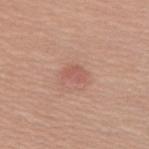| field | value |
|---|---|
| biopsy status | catalogued during a skin exam; not biopsied |
| anatomic site | the back |
| image | ~15 mm crop, total-body skin-cancer survey |
| subject | female, in their 60s |
| lighting | white-light illumination |